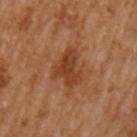Q: Was a biopsy performed?
A: total-body-photography surveillance lesion; no biopsy